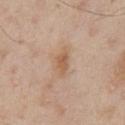Captured during whole-body skin photography for melanoma surveillance; the lesion was not biopsied. About 3 mm across. Located on the chest. A 15 mm close-up tile from a total-body photography series done for melanoma screening. A male patient in their mid- to late 60s.